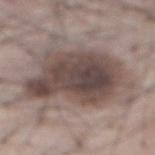image-analysis metrics = a footprint of about 55 mm², a shape eccentricity near 0.65, and two-axis asymmetry of about 0.35; roughly 13 lightness units darker than nearby skin and a normalized lesion–skin contrast near 9.5; a border-irregularity index near 7/10 and peripheral color asymmetry of about 3 | tile lighting = white-light illumination | site = the front of the torso | patient = male, aged 53–57 | diameter = about 10.5 mm | acquisition = total-body-photography crop, ~15 mm field of view.